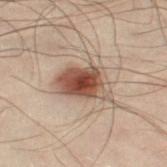workup: total-body-photography surveillance lesion; no biopsy | acquisition: total-body-photography crop, ~15 mm field of view | image-analysis metrics: a footprint of about 15 mm² and an outline eccentricity of about 0.75 (0 = round, 1 = elongated); an average lesion color of about L≈41 a*≈16 b*≈23 (CIELAB), roughly 12 lightness units darker than nearby skin, and a normalized border contrast of about 10; a border-irregularity index near 3/10, a within-lesion color-variation index near 6.5/10, and a peripheral color-asymmetry measure near 1.5; an automated nevus-likeness rating near 100 out of 100 | tile lighting: cross-polarized illumination | subject: male, about 50 years old | location: the left leg | lesion size: ≈5.5 mm.